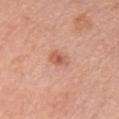– workup · no biopsy performed (imaged during a skin exam)
– patient · male, aged 58 to 62
– anatomic site · the chest
– lesion diameter · about 3 mm
– image source · ~15 mm tile from a whole-body skin photo